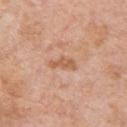Case summary:
* workup — total-body-photography surveillance lesion; no biopsy
* acquisition — ~15 mm tile from a whole-body skin photo
* anatomic site — the upper back
* patient — male, aged around 60